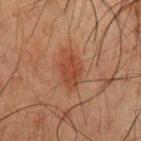The lesion was tiled from a total-body skin photograph and was not biopsied. Located on the chest. A male patient aged 48 to 52. A 15 mm close-up tile from a total-body photography series done for melanoma screening.